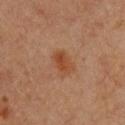{
  "biopsy_status": "not biopsied; imaged during a skin examination",
  "patient": {
    "sex": "female",
    "age_approx": 45
  },
  "lesion_size": {
    "long_diameter_mm_approx": 3.0
  },
  "lighting": "cross-polarized",
  "automated_metrics": {
    "eccentricity": 0.75,
    "shape_asymmetry": 0.15,
    "border_irregularity_0_10": 1.5,
    "color_variation_0_10": 2.5,
    "peripheral_color_asymmetry": 1.0,
    "nevus_likeness_0_100": 90,
    "lesion_detection_confidence_0_100": 100
  },
  "image": {
    "source": "total-body photography crop",
    "field_of_view_mm": 15
  },
  "site": "chest"
}